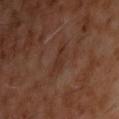  biopsy_status: not biopsied; imaged during a skin examination
  lighting: cross-polarized
  lesion_size:
    long_diameter_mm_approx: 4.0
  patient:
    sex: male
    age_approx: 60
  site: chest
  image:
    source: total-body photography crop
    field_of_view_mm: 15